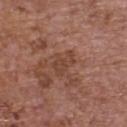Notes:
• body site: the upper back
• subject: female, in their mid- to late 60s
• acquisition: ~15 mm tile from a whole-body skin photo